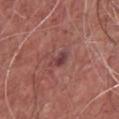biopsy_status: not biopsied; imaged during a skin examination
image:
  source: total-body photography crop
  field_of_view_mm: 15
site: chest
lesion_size:
  long_diameter_mm_approx: 2.5
patient:
  sex: male
  age_approx: 75
lighting: white-light